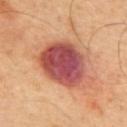follow-up = no biopsy performed (imaged during a skin exam) | site = the mid back | image source = ~15 mm crop, total-body skin-cancer survey | lighting = cross-polarized | diameter = about 6.5 mm | subject = male, aged around 55.